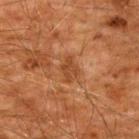Notes:
– workup — catalogued during a skin exam; not biopsied
– image source — ~15 mm crop, total-body skin-cancer survey
– site — the upper back
– image-analysis metrics — a border-irregularity rating of about 3.5/10, a color-variation rating of about 1/10, and radial color variation of about 0.5
– subject — male, approximately 60 years of age
– lighting — cross-polarized illumination